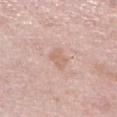| feature | finding |
|---|---|
| image | ~15 mm tile from a whole-body skin photo |
| location | the right lower leg |
| patient | female, aged approximately 50 |